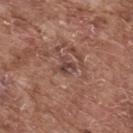Part of a total-body skin-imaging series; this lesion was reviewed on a skin check and was not flagged for biopsy. The total-body-photography lesion software estimated a lesion color around L≈43 a*≈20 b*≈23 in CIELAB, a lesion–skin lightness drop of about 8, and a normalized border contrast of about 6.5. The software also gave a border-irregularity index near 4.5/10, internal color variation of about 2 on a 0–10 scale, and peripheral color asymmetry of about 1. Captured under white-light illumination. Located on the back. Approximately 2.5 mm at its widest. Cropped from a whole-body photographic skin survey; the tile spans about 15 mm. A male patient, about 75 years old.